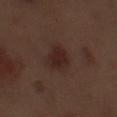Q: Is there a histopathology result?
A: no biopsy performed (imaged during a skin exam)
Q: Where on the body is the lesion?
A: the left forearm
Q: What are the patient's age and sex?
A: male, aged approximately 70
Q: How was this image acquired?
A: ~15 mm crop, total-body skin-cancer survey